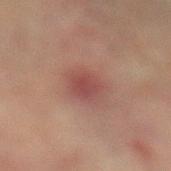biopsy_status: not biopsied; imaged during a skin examination
image:
  source: total-body photography crop
  field_of_view_mm: 15
automated_metrics:
  cielab_L: 39
  cielab_a: 22
  cielab_b: 20
  vs_skin_contrast_norm: 6.5
  border_irregularity_0_10: 1.5
  color_variation_0_10: 2.0
  peripheral_color_asymmetry: 0.5
  lesion_detection_confidence_0_100: 100
patient:
  sex: male
  age_approx: 75
lighting: cross-polarized
site: left lower leg